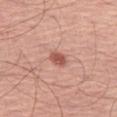Imaged during a routine full-body skin examination; the lesion was not biopsied and no histopathology is available. The total-body-photography lesion software estimated an area of roughly 4 mm², an outline eccentricity of about 0.65 (0 = round, 1 = elongated), and a symmetry-axis asymmetry near 0.25. It also reported roughly 12 lightness units darker than nearby skin and a lesion-to-skin contrast of about 8 (normalized; higher = more distinct). The software also gave lesion-presence confidence of about 100/100. From the left thigh. A roughly 15 mm field-of-view crop from a total-body skin photograph. The patient is a male aged 63–67. This is a white-light tile. Longest diameter approximately 2.5 mm.